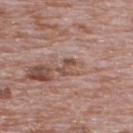Impression:
The lesion was photographed on a routine skin check and not biopsied; there is no pathology result.
Acquisition and patient details:
Imaged with white-light lighting. The total-body-photography lesion software estimated border irregularity of about 4 on a 0–10 scale, a color-variation rating of about 0/10, and a peripheral color-asymmetry measure near 0. It also reported an automated nevus-likeness rating near 0 out of 100 and lesion-presence confidence of about 90/100. From the upper back. The recorded lesion diameter is about 2.5 mm. The patient is a male aged 68–72. A 15 mm close-up extracted from a 3D total-body photography capture.